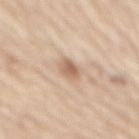No biopsy was performed on this lesion — it was imaged during a full skin examination and was not determined to be concerning. The subject is a female approximately 65 years of age. A 15 mm close-up tile from a total-body photography series done for melanoma screening. The lesion-visualizer software estimated a footprint of about 3.5 mm², a shape eccentricity near 0.85, and two-axis asymmetry of about 0.4. It also reported an average lesion color of about L≈62 a*≈17 b*≈31 (CIELAB), a lesion–skin lightness drop of about 12, and a normalized lesion–skin contrast near 7.5. The analysis additionally found a classifier nevus-likeness of about 55/100 and a lesion-detection confidence of about 100/100. The lesion is located on the mid back. Measured at roughly 3 mm in maximum diameter.